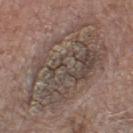Imaged during a routine full-body skin examination; the lesion was not biopsied and no histopathology is available.
Measured at roughly 13.5 mm in maximum diameter.
An algorithmic analysis of the crop reported an average lesion color of about L≈47 a*≈12 b*≈21 (CIELAB), a lesion–skin lightness drop of about 7, and a normalized lesion–skin contrast near 6. And it measured a border-irregularity index near 4.5/10. The software also gave a classifier nevus-likeness of about 0/100.
The patient is a male aged around 75.
The lesion is on the leg.
A lesion tile, about 15 mm wide, cut from a 3D total-body photograph.